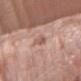Assessment: Captured during whole-body skin photography for melanoma surveillance; the lesion was not biopsied. Clinical summary: A male patient about 75 years old. The lesion is on the left forearm. A close-up tile cropped from a whole-body skin photograph, about 15 mm across.